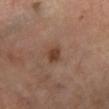- workup · imaged on a skin check; not biopsied